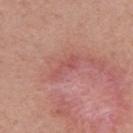Impression: Imaged during a routine full-body skin examination; the lesion was not biopsied and no histopathology is available. Context: A 15 mm crop from a total-body photograph taken for skin-cancer surveillance. The lesion is located on the left upper arm. A female subject, approximately 55 years of age.